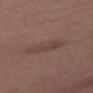Imaged during a routine full-body skin examination; the lesion was not biopsied and no histopathology is available. Measured at roughly 5 mm in maximum diameter. The lesion is on the right thigh. A female patient, roughly 50 years of age. Captured under cross-polarized illumination. A region of skin cropped from a whole-body photographic capture, roughly 15 mm wide.